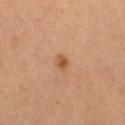Image and clinical context: The tile uses cross-polarized illumination. Measured at roughly 2 mm in maximum diameter. The lesion is located on the right thigh. A lesion tile, about 15 mm wide, cut from a 3D total-body photograph. A female subject aged 53 to 57.